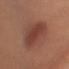workup = imaged on a skin check; not biopsied | imaging modality = ~15 mm crop, total-body skin-cancer survey | image-analysis metrics = a border-irregularity rating of about 3/10 and radial color variation of about 1.5 | size = ~8.5 mm (longest diameter) | lighting = white-light | location = the left upper arm | patient = female, aged approximately 55.